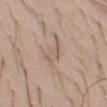No biopsy was performed on this lesion — it was imaged during a full skin examination and was not determined to be concerning.